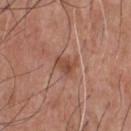Captured during whole-body skin photography for melanoma surveillance; the lesion was not biopsied.
The lesion-visualizer software estimated an average lesion color of about L≈47 a*≈23 b*≈29 (CIELAB), a lesion–skin lightness drop of about 9, and a lesion-to-skin contrast of about 7 (normalized; higher = more distinct). It also reported a border-irregularity index near 3/10, internal color variation of about 3 on a 0–10 scale, and peripheral color asymmetry of about 1. The analysis additionally found a detector confidence of about 100 out of 100 that the crop contains a lesion.
A male subject about 70 years old.
Measured at roughly 2.5 mm in maximum diameter.
A region of skin cropped from a whole-body photographic capture, roughly 15 mm wide.
The lesion is on the chest.